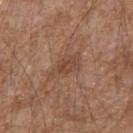Clinical impression: Recorded during total-body skin imaging; not selected for excision or biopsy. Clinical summary: Imaged with white-light lighting. A male patient aged 53–57. Cropped from a whole-body photographic skin survey; the tile spans about 15 mm. Located on the right upper arm. The lesion-visualizer software estimated a lesion area of about 7 mm², a shape eccentricity near 0.9, and a shape-asymmetry score of about 0.3 (0 = symmetric). And it measured an average lesion color of about L≈46 a*≈20 b*≈29 (CIELAB) and about 7 CIELAB-L* units darker than the surrounding skin.